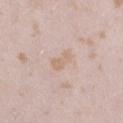biopsy_status: not biopsied; imaged during a skin examination
site: right thigh
patient:
  sex: female
  age_approx: 25
image:
  source: total-body photography crop
  field_of_view_mm: 15